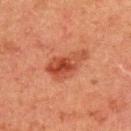{
  "lesion_size": {
    "long_diameter_mm_approx": 5.5
  },
  "image": {
    "source": "total-body photography crop",
    "field_of_view_mm": 15
  },
  "patient": {
    "sex": "male",
    "age_approx": 50
  },
  "lighting": "cross-polarized",
  "site": "upper back",
  "automated_metrics": {
    "cielab_L": 38,
    "cielab_a": 26,
    "cielab_b": 30,
    "lesion_detection_confidence_0_100": 100
  }
}